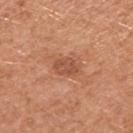The tile uses white-light illumination. This image is a 15 mm lesion crop taken from a total-body photograph. The lesion is on the right upper arm. A female subject in their 40s. Approximately 3 mm at its widest.